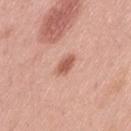follow-up: no biopsy performed (imaged during a skin exam)
body site: the lower back
lesion diameter: ~3 mm (longest diameter)
image source: ~15 mm crop, total-body skin-cancer survey
lighting: white-light
subject: female, about 40 years old